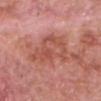Clinical impression: No biopsy was performed on this lesion — it was imaged during a full skin examination and was not determined to be concerning.